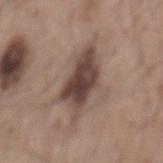follow-up=total-body-photography surveillance lesion; no biopsy
size=~7 mm (longest diameter)
patient=male, approximately 55 years of age
anatomic site=the mid back
image source=~15 mm tile from a whole-body skin photo